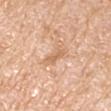Q: Is there a histopathology result?
A: imaged on a skin check; not biopsied
Q: How was the tile lit?
A: white-light
Q: Automated lesion metrics?
A: a footprint of about 5 mm², an outline eccentricity of about 0.9 (0 = round, 1 = elongated), and a symmetry-axis asymmetry near 0.25
Q: How was this image acquired?
A: 15 mm crop, total-body photography
Q: Lesion size?
A: ≈3.5 mm
Q: Who is the patient?
A: male, approximately 60 years of age
Q: What is the anatomic site?
A: the right upper arm